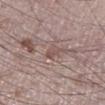{
  "lighting": "white-light",
  "automated_metrics": {
    "vs_skin_darker_L": 7.0,
    "vs_skin_contrast_norm": 5.5,
    "color_variation_0_10": 1.0,
    "peripheral_color_asymmetry": 0.0
  },
  "site": "left lower leg",
  "patient": {
    "sex": "male",
    "age_approx": 75
  },
  "lesion_size": {
    "long_diameter_mm_approx": 3.0
  },
  "image": {
    "source": "total-body photography crop",
    "field_of_view_mm": 15
  }
}